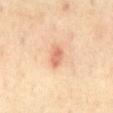tile lighting = cross-polarized illumination | image = ~15 mm crop, total-body skin-cancer survey | site = the abdomen | size = ~2.5 mm (longest diameter) | TBP lesion metrics = an eccentricity of roughly 0.7 and two-axis asymmetry of about 0.2; a within-lesion color-variation index near 3/10 and a peripheral color-asymmetry measure near 1; a nevus-likeness score of about 25/100 and a detector confidence of about 100 out of 100 that the crop contains a lesion | patient = male, roughly 65 years of age.